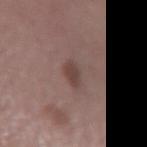<record>
<biopsy_status>not biopsied; imaged during a skin examination</biopsy_status>
<site>left forearm</site>
<image>
  <source>total-body photography crop</source>
  <field_of_view_mm>15</field_of_view_mm>
</image>
<lighting>white-light</lighting>
<patient>
  <sex>female</sex>
  <age_approx>50</age_approx>
</patient>
</record>